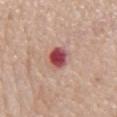<lesion>
<image>
  <source>total-body photography crop</source>
  <field_of_view_mm>15</field_of_view_mm>
</image>
<patient>
  <sex>male</sex>
  <age_approx>75</age_approx>
</patient>
<lesion_size>
  <long_diameter_mm_approx>3.0</long_diameter_mm_approx>
</lesion_size>
<automated_metrics>
  <area_mm2_approx>6.0</area_mm2_approx>
  <eccentricity>0.65</eccentricity>
  <shape_asymmetry>0.1</shape_asymmetry>
  <cielab_L>49</cielab_L>
  <cielab_a>32</cielab_a>
  <cielab_b>23</cielab_b>
  <vs_skin_darker_L>17.0</vs_skin_darker_L>
</automated_metrics>
<site>chest</site>
<lighting>white-light</lighting>
</lesion>